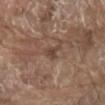Assessment: Captured during whole-body skin photography for melanoma surveillance; the lesion was not biopsied. Background: Located on the abdomen. This image is a 15 mm lesion crop taken from a total-body photograph. A male patient about 80 years old. Imaged with white-light lighting.